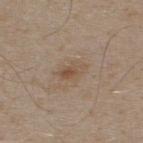<case>
<biopsy_status>not biopsied; imaged during a skin examination</biopsy_status>
<image>
  <source>total-body photography crop</source>
  <field_of_view_mm>15</field_of_view_mm>
</image>
<automated_metrics>
  <area_mm2_approx>5.0</area_mm2_approx>
  <eccentricity>0.85</eccentricity>
  <shape_asymmetry>0.2</shape_asymmetry>
  <cielab_L>51</cielab_L>
  <cielab_a>14</cielab_a>
  <cielab_b>28</cielab_b>
  <vs_skin_darker_L>7.0</vs_skin_darker_L>
  <vs_skin_contrast_norm>6.0</vs_skin_contrast_norm>
  <nevus_likeness_0_100>5</nevus_likeness_0_100>
  <lesion_detection_confidence_0_100>100</lesion_detection_confidence_0_100>
</automated_metrics>
<patient>
  <sex>male</sex>
  <age_approx>30</age_approx>
</patient>
<lighting>white-light</lighting>
<site>upper back</site>
</case>